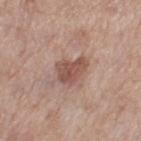No biopsy was performed on this lesion — it was imaged during a full skin examination and was not determined to be concerning. The lesion's longest dimension is about 3.5 mm. A female patient roughly 55 years of age. The lesion is on the leg. A roughly 15 mm field-of-view crop from a total-body skin photograph. Automated tile analysis of the lesion measured a footprint of about 8 mm², an outline eccentricity of about 0.65 (0 = round, 1 = elongated), and a shape-asymmetry score of about 0.3 (0 = symmetric). And it measured an average lesion color of about L≈52 a*≈21 b*≈25 (CIELAB), about 11 CIELAB-L* units darker than the surrounding skin, and a normalized lesion–skin contrast near 7.5. And it measured a border-irregularity index near 3/10, internal color variation of about 3 on a 0–10 scale, and radial color variation of about 1. The software also gave a nevus-likeness score of about 60/100 and a lesion-detection confidence of about 100/100. Captured under white-light illumination.